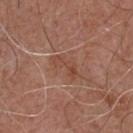Captured during whole-body skin photography for melanoma surveillance; the lesion was not biopsied.